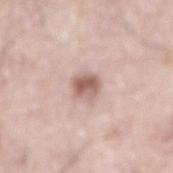• follow-up — total-body-photography surveillance lesion; no biopsy
• lesion diameter — about 3 mm
• subject — male, roughly 75 years of age
• acquisition — 15 mm crop, total-body photography
• tile lighting — white-light
• body site — the abdomen